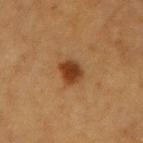Captured during whole-body skin photography for melanoma surveillance; the lesion was not biopsied. A female subject about 55 years old. From the left forearm. Imaged with cross-polarized lighting. Measured at roughly 3 mm in maximum diameter. A 15 mm crop from a total-body photograph taken for skin-cancer surveillance.